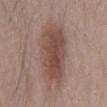Recorded during total-body skin imaging; not selected for excision or biopsy. This is a white-light tile. On the mid back. The subject is a male approximately 45 years of age. A roughly 15 mm field-of-view crop from a total-body skin photograph.